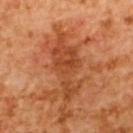{
  "biopsy_status": "not biopsied; imaged during a skin examination",
  "image": {
    "source": "total-body photography crop",
    "field_of_view_mm": 15
  },
  "automated_metrics": {
    "area_mm2_approx": 16.0,
    "eccentricity": 0.9
  },
  "site": "upper back",
  "lesion_size": {
    "long_diameter_mm_approx": 7.5
  },
  "lighting": "cross-polarized",
  "patient": {
    "sex": "female",
    "age_approx": 55
  }
}